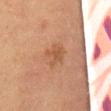Imaged during a routine full-body skin examination; the lesion was not biopsied and no histopathology is available. Located on the abdomen. The tile uses cross-polarized illumination. The lesion-visualizer software estimated a border-irregularity index near 5/10, internal color variation of about 1 on a 0–10 scale, and a peripheral color-asymmetry measure near 0. The software also gave a nevus-likeness score of about 5/100 and a detector confidence of about 100 out of 100 that the crop contains a lesion. Approximately 2.5 mm at its widest. A female subject, approximately 50 years of age. A lesion tile, about 15 mm wide, cut from a 3D total-body photograph.